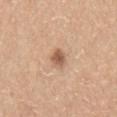Part of a total-body skin-imaging series; this lesion was reviewed on a skin check and was not flagged for biopsy. This image is a 15 mm lesion crop taken from a total-body photograph. This is a white-light tile. A male patient, about 20 years old. The total-body-photography lesion software estimated a mean CIELAB color near L≈57 a*≈21 b*≈31 and roughly 13 lightness units darker than nearby skin. Located on the front of the torso. Measured at roughly 2.5 mm in maximum diameter.